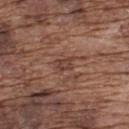The lesion was tiled from a total-body skin photograph and was not biopsied.
The lesion's longest dimension is about 2.5 mm.
Located on the upper back.
Imaged with white-light lighting.
The patient is a male aged approximately 75.
A 15 mm close-up tile from a total-body photography series done for melanoma screening.